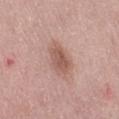| key | value |
|---|---|
| notes | catalogued during a skin exam; not biopsied |
| image | ~15 mm tile from a whole-body skin photo |
| location | the right thigh |
| patient | female, aged around 50 |